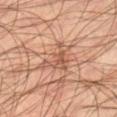Q: Is there a histopathology result?
A: imaged on a skin check; not biopsied
Q: How large is the lesion?
A: about 4 mm
Q: What are the patient's age and sex?
A: male, in their mid- to late 40s
Q: What lighting was used for the tile?
A: cross-polarized
Q: Where on the body is the lesion?
A: the right lower leg
Q: How was this image acquired?
A: ~15 mm tile from a whole-body skin photo